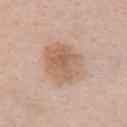No biopsy was performed on this lesion — it was imaged during a full skin examination and was not determined to be concerning. A female subject, aged around 40. Automated tile analysis of the lesion measured border irregularity of about 1.5 on a 0–10 scale, a within-lesion color-variation index near 4.5/10, and peripheral color asymmetry of about 1.5. Located on the chest. This is a white-light tile. A close-up tile cropped from a whole-body skin photograph, about 15 mm across. Measured at roughly 6 mm in maximum diameter.